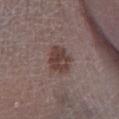Case summary:
- workup — catalogued during a skin exam; not biopsied
- diameter — ≈4 mm
- illumination — white-light
- patient — male, approximately 70 years of age
- location — the left lower leg
- image — 15 mm crop, total-body photography